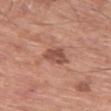Assessment: Imaged during a routine full-body skin examination; the lesion was not biopsied and no histopathology is available. Clinical summary: On the leg. A region of skin cropped from a whole-body photographic capture, roughly 15 mm wide. This is a white-light tile. The patient is a male in their 60s. The recorded lesion diameter is about 3 mm.